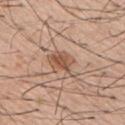Clinical impression: Captured during whole-body skin photography for melanoma surveillance; the lesion was not biopsied. Context: A region of skin cropped from a whole-body photographic capture, roughly 15 mm wide. The subject is a male about 55 years old. The lesion-visualizer software estimated a lesion area of about 7.5 mm², a shape eccentricity near 0.8, and two-axis asymmetry of about 0.5. The software also gave roughly 10 lightness units darker than nearby skin and a normalized lesion–skin contrast near 7. And it measured a color-variation rating of about 4/10 and radial color variation of about 1.5.